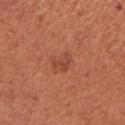Findings:
• notes · total-body-photography surveillance lesion; no biopsy
• image-analysis metrics · a shape eccentricity near 0.75; border irregularity of about 4 on a 0–10 scale and peripheral color asymmetry of about 1
• lesion diameter · ≈2.5 mm
• location · the right upper arm
• subject · female, in their mid-60s
• illumination · white-light illumination
• image · ~15 mm crop, total-body skin-cancer survey